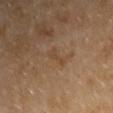Impression: No biopsy was performed on this lesion — it was imaged during a full skin examination and was not determined to be concerning. Image and clinical context: A 15 mm close-up extracted from a 3D total-body photography capture. The lesion is located on the right upper arm. Approximately 3 mm at its widest. A male patient, in their mid-50s. The total-body-photography lesion software estimated an area of roughly 2.5 mm², an eccentricity of roughly 0.9, and two-axis asymmetry of about 0.65. The analysis additionally found an average lesion color of about L≈40 a*≈16 b*≈30 (CIELAB) and a normalized lesion–skin contrast near 5.